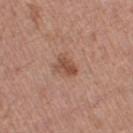biopsy status=total-body-photography surveillance lesion; no biopsy | size=about 2.5 mm | patient=female, aged 73–77 | anatomic site=the left thigh | imaging modality=total-body-photography crop, ~15 mm field of view.